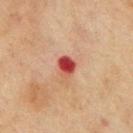Q: Is there a histopathology result?
A: no biopsy performed (imaged during a skin exam)
Q: Lesion location?
A: the mid back
Q: Who is the patient?
A: male, aged approximately 70
Q: How was this image acquired?
A: total-body-photography crop, ~15 mm field of view
Q: How large is the lesion?
A: about 2.5 mm